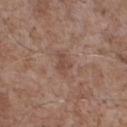Notes:
– follow-up: no biopsy performed (imaged during a skin exam)
– image-analysis metrics: an average lesion color of about L≈48 a*≈19 b*≈26 (CIELAB), a lesion–skin lightness drop of about 7, and a normalized border contrast of about 5.5; a peripheral color-asymmetry measure near 0.5; a classifier nevus-likeness of about 0/100
– imaging modality: 15 mm crop, total-body photography
– patient: male, aged approximately 70
– body site: the chest
– illumination: white-light illumination
– diameter: ≈3 mm